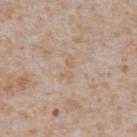follow-up — imaged on a skin check; not biopsied
automated lesion analysis — a lesion area of about 3 mm², an eccentricity of roughly 0.9, and a symmetry-axis asymmetry near 0.4; a lesion color around L≈62 a*≈16 b*≈30 in CIELAB and a lesion–skin lightness drop of about 5; a border-irregularity rating of about 5/10, a within-lesion color-variation index near 0/10, and a peripheral color-asymmetry measure near 0; a classifier nevus-likeness of about 0/100
subject — male, in their mid-60s
tile lighting — white-light illumination
image source — 15 mm crop, total-body photography
site — the abdomen